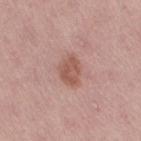{"biopsy_status": "not biopsied; imaged during a skin examination", "image": {"source": "total-body photography crop", "field_of_view_mm": 15}, "site": "right thigh", "patient": {"sex": "female", "age_approx": 50}}